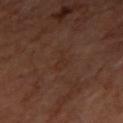Clinical impression: The lesion was tiled from a total-body skin photograph and was not biopsied. Acquisition and patient details: The tile uses cross-polarized illumination. Longest diameter approximately 2.5 mm. The lesion is on the arm. A region of skin cropped from a whole-body photographic capture, roughly 15 mm wide. The patient is a male aged approximately 65.